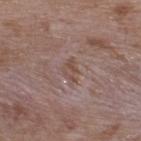* workup · no biopsy performed (imaged during a skin exam)
* image · total-body-photography crop, ~15 mm field of view
* patient · male, aged 48–52
* tile lighting · white-light illumination
* body site · the upper back